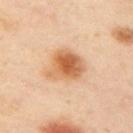The lesion was tiled from a total-body skin photograph and was not biopsied.
Approximately 5 mm at its widest.
The lesion is on the arm.
Automated image analysis of the tile measured an average lesion color of about L≈65 a*≈24 b*≈40 (CIELAB) and roughly 14 lightness units darker than nearby skin.
Captured under cross-polarized illumination.
Cropped from a whole-body photographic skin survey; the tile spans about 15 mm.
A female patient approximately 40 years of age.